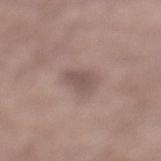Impression: Imaged during a routine full-body skin examination; the lesion was not biopsied and no histopathology is available. Clinical summary: The lesion is located on the leg. A male patient roughly 55 years of age. A 15 mm crop from a total-body photograph taken for skin-cancer surveillance. An algorithmic analysis of the crop reported a mean CIELAB color near L≈51 a*≈15 b*≈19, a lesion–skin lightness drop of about 9, and a lesion-to-skin contrast of about 6.5 (normalized; higher = more distinct).